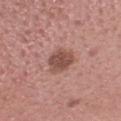  biopsy_status: not biopsied; imaged during a skin examination
  patient:
    sex: female
    age_approx: 20
  site: head or neck
  lesion_size:
    long_diameter_mm_approx: 3.5
  image:
    source: total-body photography crop
    field_of_view_mm: 15